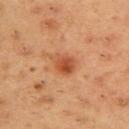workup: imaged on a skin check; not biopsied
lighting: cross-polarized
automated lesion analysis: a mean CIELAB color near L≈51 a*≈28 b*≈38, roughly 11 lightness units darker than nearby skin, and a normalized border contrast of about 7.5
site: the upper back
acquisition: ~15 mm tile from a whole-body skin photo
lesion diameter: about 3.5 mm
patient: male, aged 53 to 57